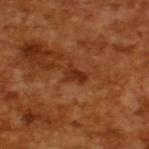This lesion was catalogued during total-body skin photography and was not selected for biopsy.